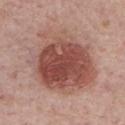notes — imaged on a skin check; not biopsied | location — the chest | imaging modality — ~15 mm crop, total-body skin-cancer survey | diameter — ≈9.5 mm | patient — male, aged 73–77 | automated lesion analysis — a mean CIELAB color near L≈50 a*≈23 b*≈25 and about 13 CIELAB-L* units darker than the surrounding skin; a nevus-likeness score of about 85/100 and a lesion-detection confidence of about 100/100 | lighting — white-light illumination.